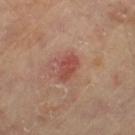  biopsy_status: not biopsied; imaged during a skin examination
  automated_metrics:
    area_mm2_approx: 5.5
    shape_asymmetry: 0.2
    vs_skin_darker_L: 9.0
    vs_skin_contrast_norm: 7.0
  site: left thigh
  patient:
    sex: male
    age_approx: 65
  lighting: cross-polarized
  image:
    source: total-body photography crop
    field_of_view_mm: 15
  lesion_size:
    long_diameter_mm_approx: 3.5